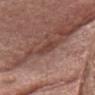The lesion was tiled from a total-body skin photograph and was not biopsied. The lesion-visualizer software estimated a lesion area of about 3.5 mm², a shape eccentricity near 0.9, and a symmetry-axis asymmetry near 0.25. And it measured an average lesion color of about L≈41 a*≈22 b*≈24 (CIELAB) and a lesion–skin lightness drop of about 7. The software also gave border irregularity of about 3 on a 0–10 scale, internal color variation of about 1.5 on a 0–10 scale, and radial color variation of about 0.5. The lesion is on the head or neck. Cropped from a total-body skin-imaging series; the visible field is about 15 mm. A female patient aged approximately 65. Measured at roughly 3 mm in maximum diameter. The tile uses white-light illumination.